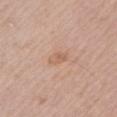Image and clinical context: On the left upper arm. Imaged with white-light lighting. This image is a 15 mm lesion crop taken from a total-body photograph. The subject is a male aged approximately 65. Measured at roughly 3 mm in maximum diameter.